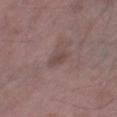<tbp_lesion>
<biopsy_status>not biopsied; imaged during a skin examination</biopsy_status>
<lighting>white-light</lighting>
<automated_metrics>
  <border_irregularity_0_10>2.0</border_irregularity_0_10>
  <color_variation_0_10>1.5</color_variation_0_10>
  <peripheral_color_asymmetry>0.5</peripheral_color_asymmetry>
  <nevus_likeness_0_100>0</nevus_likeness_0_100>
  <lesion_detection_confidence_0_100>90</lesion_detection_confidence_0_100>
</automated_metrics>
<lesion_size>
  <long_diameter_mm_approx>2.5</long_diameter_mm_approx>
</lesion_size>
<patient>
  <sex>male</sex>
  <age_approx>50</age_approx>
</patient>
<site>right thigh</site>
<image>
  <source>total-body photography crop</source>
  <field_of_view_mm>15</field_of_view_mm>
</image>
</tbp_lesion>